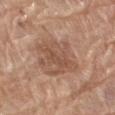An algorithmic analysis of the crop reported an area of roughly 13 mm² and a shape-asymmetry score of about 0.3 (0 = symmetric).
The tile uses white-light illumination.
A female patient roughly 75 years of age.
A region of skin cropped from a whole-body photographic capture, roughly 15 mm wide.
Longest diameter approximately 4.5 mm.
The lesion is located on the left thigh.